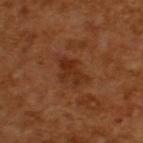Clinical impression:
Part of a total-body skin-imaging series; this lesion was reviewed on a skin check and was not flagged for biopsy.
Context:
The subject is a male aged approximately 65. The tile uses cross-polarized illumination. A 15 mm close-up tile from a total-body photography series done for melanoma screening.